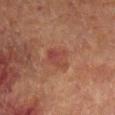Assessment: Imaged during a routine full-body skin examination; the lesion was not biopsied and no histopathology is available. Acquisition and patient details: On the leg. A 15 mm close-up extracted from a 3D total-body photography capture. Imaged with cross-polarized lighting. Measured at roughly 3.5 mm in maximum diameter. A male patient aged 68–72. The lesion-visualizer software estimated a lesion color around L≈36 a*≈22 b*≈23 in CIELAB, a lesion–skin lightness drop of about 6, and a lesion-to-skin contrast of about 6 (normalized; higher = more distinct). The software also gave a color-variation rating of about 4.5/10 and a peripheral color-asymmetry measure near 1.5. The analysis additionally found an automated nevus-likeness rating near 30 out of 100.